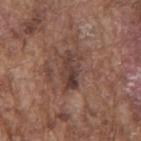Q: Was a biopsy performed?
A: total-body-photography surveillance lesion; no biopsy
Q: What are the patient's age and sex?
A: male, approximately 75 years of age
Q: What lighting was used for the tile?
A: white-light
Q: What kind of image is this?
A: total-body-photography crop, ~15 mm field of view
Q: Where on the body is the lesion?
A: the back